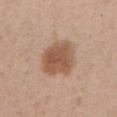Impression: This lesion was catalogued during total-body skin photography and was not selected for biopsy.